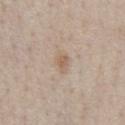Impression: Captured during whole-body skin photography for melanoma surveillance; the lesion was not biopsied. Acquisition and patient details: A male subject in their 60s. The tile uses white-light illumination. The lesion is on the front of the torso. A roughly 15 mm field-of-view crop from a total-body skin photograph.